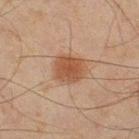Captured during whole-body skin photography for melanoma surveillance; the lesion was not biopsied. Imaged with cross-polarized lighting. A 15 mm close-up extracted from a 3D total-body photography capture. Measured at roughly 3.5 mm in maximum diameter. From the right thigh. The patient is a male about 45 years old.